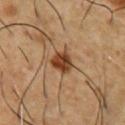This lesion was catalogued during total-body skin photography and was not selected for biopsy. From the chest. A 15 mm crop from a total-body photograph taken for skin-cancer surveillance. The total-body-photography lesion software estimated an area of roughly 6 mm², a shape eccentricity near 0.55, and a shape-asymmetry score of about 0.25 (0 = symmetric). The analysis additionally found an automated nevus-likeness rating near 95 out of 100 and a lesion-detection confidence of about 100/100. A male patient, approximately 55 years of age. Imaged with cross-polarized lighting.